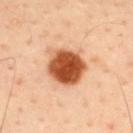site: mid back
lesion_size:
  long_diameter_mm_approx: 5.0
patient:
  sex: male
  age_approx: 45
automated_metrics:
  cielab_L: 54
  cielab_a: 29
  cielab_b: 40
  vs_skin_darker_L: 21.0
  lesion_detection_confidence_0_100: 100
image:
  source: total-body photography crop
  field_of_view_mm: 15
lighting: cross-polarized